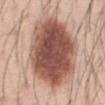Clinical impression: Captured during whole-body skin photography for melanoma surveillance; the lesion was not biopsied. Context: The lesion's longest dimension is about 9.5 mm. An algorithmic analysis of the crop reported an area of roughly 55 mm², a shape eccentricity near 0.7, and two-axis asymmetry of about 0.1. It also reported a mean CIELAB color near L≈52 a*≈22 b*≈27, about 19 CIELAB-L* units darker than the surrounding skin, and a lesion-to-skin contrast of about 12 (normalized; higher = more distinct). The software also gave a nevus-likeness score of about 95/100 and lesion-presence confidence of about 100/100. From the abdomen. A male subject, aged 43 to 47. Cropped from a total-body skin-imaging series; the visible field is about 15 mm.